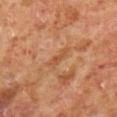No biopsy was performed on this lesion — it was imaged during a full skin examination and was not determined to be concerning.